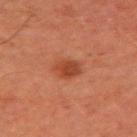Q: Was this lesion biopsied?
A: total-body-photography surveillance lesion; no biopsy
Q: How was the tile lit?
A: cross-polarized
Q: What is the anatomic site?
A: the arm
Q: What are the patient's age and sex?
A: male, about 65 years old
Q: Lesion size?
A: ~3 mm (longest diameter)
Q: How was this image acquired?
A: 15 mm crop, total-body photography